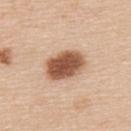Findings:
– notes — no biopsy performed (imaged during a skin exam)
– anatomic site — the upper back
– image source — ~15 mm crop, total-body skin-cancer survey
– image-analysis metrics — an area of roughly 13 mm², an eccentricity of roughly 0.75, and a shape-asymmetry score of about 0.1 (0 = symmetric); a mean CIELAB color near L≈55 a*≈22 b*≈32, about 19 CIELAB-L* units darker than the surrounding skin, and a normalized border contrast of about 12; border irregularity of about 1 on a 0–10 scale, a color-variation rating of about 4.5/10, and peripheral color asymmetry of about 1.5; a nevus-likeness score of about 100/100 and lesion-presence confidence of about 100/100
– lesion diameter — about 4.5 mm
– patient — female, aged around 45